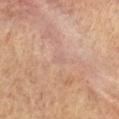No biopsy was performed on this lesion — it was imaged during a full skin examination and was not determined to be concerning. The lesion is located on the chest. Measured at roughly 1.5 mm in maximum diameter. Captured under cross-polarized illumination. This image is a 15 mm lesion crop taken from a total-body photograph. The subject is a female about 80 years old.